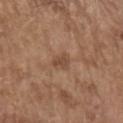No biopsy was performed on this lesion — it was imaged during a full skin examination and was not determined to be concerning.
A 15 mm close-up extracted from a 3D total-body photography capture.
The lesion-visualizer software estimated a mean CIELAB color near L≈47 a*≈19 b*≈29, about 8 CIELAB-L* units darker than the surrounding skin, and a normalized border contrast of about 6.5. The software also gave a within-lesion color-variation index near 0.5/10.
On the left upper arm.
Imaged with white-light lighting.
A male patient, approximately 80 years of age.